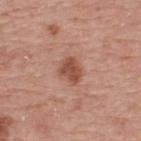Part of a total-body skin-imaging series; this lesion was reviewed on a skin check and was not flagged for biopsy. The lesion-visualizer software estimated an area of roughly 6 mm² and an eccentricity of roughly 0.65. And it measured a lesion color around L≈50 a*≈25 b*≈29 in CIELAB, roughly 11 lightness units darker than nearby skin, and a normalized lesion–skin contrast near 8. It also reported a nevus-likeness score of about 85/100 and lesion-presence confidence of about 100/100. A 15 mm close-up extracted from a 3D total-body photography capture. The tile uses white-light illumination. The lesion's longest dimension is about 3 mm. The lesion is on the back. A male patient roughly 75 years of age.